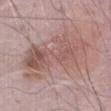The lesion was tiled from a total-body skin photograph and was not biopsied. Measured at roughly 10.5 mm in maximum diameter. A male patient in their mid- to late 70s. A 15 mm close-up extracted from a 3D total-body photography capture. The lesion is on the abdomen. An algorithmic analysis of the crop reported a lesion area of about 35 mm², a shape eccentricity near 0.9, and two-axis asymmetry of about 0.25. It also reported a border-irregularity index near 5.5/10 and radial color variation of about 2.5. The analysis additionally found an automated nevus-likeness rating near 0 out of 100 and lesion-presence confidence of about 95/100. Imaged with white-light lighting.